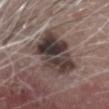No biopsy was performed on this lesion — it was imaged during a full skin examination and was not determined to be concerning. The lesion is on the head or neck. The patient is a male aged 78 to 82. About 7 mm across. Imaged with white-light lighting. This image is a 15 mm lesion crop taken from a total-body photograph.